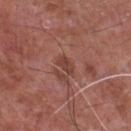Clinical impression: Part of a total-body skin-imaging series; this lesion was reviewed on a skin check and was not flagged for biopsy. Image and clinical context: Captured under white-light illumination. From the chest. The lesion's longest dimension is about 2.5 mm. A male patient, in their mid- to late 60s. An algorithmic analysis of the crop reported an area of roughly 3 mm², an outline eccentricity of about 0.85 (0 = round, 1 = elongated), and two-axis asymmetry of about 0.25. And it measured a lesion color around L≈42 a*≈24 b*≈26 in CIELAB, a lesion–skin lightness drop of about 8, and a normalized lesion–skin contrast near 6.5. It also reported a border-irregularity rating of about 2.5/10, a color-variation rating of about 1/10, and radial color variation of about 0.5. And it measured a classifier nevus-likeness of about 0/100. A 15 mm crop from a total-body photograph taken for skin-cancer surveillance.